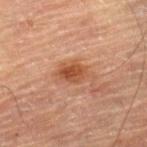follow-up — no biopsy performed (imaged during a skin exam); diameter — about 4.5 mm; anatomic site — the right lower leg; subject — male, about 85 years old or older; image source — 15 mm crop, total-body photography; tile lighting — cross-polarized.